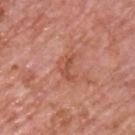Q: Was a biopsy performed?
A: catalogued during a skin exam; not biopsied
Q: Illumination type?
A: white-light
Q: What is the anatomic site?
A: the chest
Q: What kind of image is this?
A: 15 mm crop, total-body photography
Q: Patient demographics?
A: male, in their mid- to late 70s